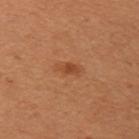| key | value |
|---|---|
| biopsy status | catalogued during a skin exam; not biopsied |
| patient | female, in their 50s |
| anatomic site | the left upper arm |
| imaging modality | ~15 mm tile from a whole-body skin photo |